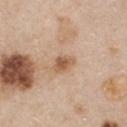Background:
Automated tile analysis of the lesion measured a lesion area of about 4.5 mm², an outline eccentricity of about 0.75 (0 = round, 1 = elongated), and a shape-asymmetry score of about 0.25 (0 = symmetric). And it measured a border-irregularity index near 2/10, a within-lesion color-variation index near 3/10, and peripheral color asymmetry of about 1. The software also gave a classifier nevus-likeness of about 45/100 and a detector confidence of about 100 out of 100 that the crop contains a lesion. A female subject, aged 43–47. Measured at roughly 2.5 mm in maximum diameter. A close-up tile cropped from a whole-body skin photograph, about 15 mm across. On the chest. Imaged with white-light lighting.